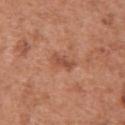Impression:
This lesion was catalogued during total-body skin photography and was not selected for biopsy.
Context:
The recorded lesion diameter is about 3 mm. Captured under white-light illumination. A male patient, aged 63 to 67. A roughly 15 mm field-of-view crop from a total-body skin photograph. Located on the chest.